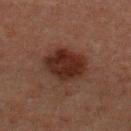Q: Is there a histopathology result?
A: total-body-photography surveillance lesion; no biopsy